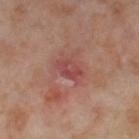Acquisition and patient details: Located on the right thigh. A region of skin cropped from a whole-body photographic capture, roughly 15 mm wide. The patient is a female roughly 55 years of age.